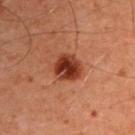follow-up — imaged on a skin check; not biopsied | location — the left upper arm | subject — male, aged 58–62 | acquisition — 15 mm crop, total-body photography.